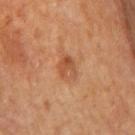Q: Was a biopsy performed?
A: imaged on a skin check; not biopsied
Q: What kind of image is this?
A: ~15 mm tile from a whole-body skin photo
Q: Who is the patient?
A: male, aged 63 to 67
Q: How was the tile lit?
A: cross-polarized
Q: Where on the body is the lesion?
A: the mid back